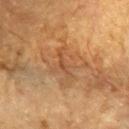Clinical impression: No biopsy was performed on this lesion — it was imaged during a full skin examination and was not determined to be concerning. Acquisition and patient details: A male subject, in their mid-80s or older. An algorithmic analysis of the crop reported an area of roughly 17 mm². It also reported a color-variation rating of about 4.5/10 and peripheral color asymmetry of about 1.5. The analysis additionally found a nevus-likeness score of about 0/100 and a detector confidence of about 55 out of 100 that the crop contains a lesion. Cropped from a whole-body photographic skin survey; the tile spans about 15 mm. This is a cross-polarized tile. From the head or neck.